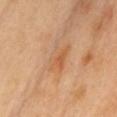{"biopsy_status": "not biopsied; imaged during a skin examination", "site": "chest", "lesion_size": {"long_diameter_mm_approx": 4.0}, "automated_metrics": {"area_mm2_approx": 8.0, "eccentricity": 0.85, "shape_asymmetry": 0.25, "cielab_L": 60, "cielab_a": 23, "cielab_b": 38, "vs_skin_darker_L": 7.0, "vs_skin_contrast_norm": 5.0, "border_irregularity_0_10": 3.0, "color_variation_0_10": 4.5, "peripheral_color_asymmetry": 1.5, "nevus_likeness_0_100": 0, "lesion_detection_confidence_0_100": 100}, "image": {"source": "total-body photography crop", "field_of_view_mm": 15}, "lighting": "cross-polarized", "patient": {"sex": "female", "age_approx": 40}}